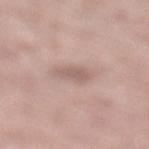biopsy status = imaged on a skin check; not biopsied
image source = ~15 mm tile from a whole-body skin photo
anatomic site = the left lower leg
patient = male, in their mid- to late 50s